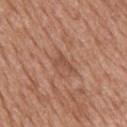<record>
<biopsy_status>not biopsied; imaged during a skin examination</biopsy_status>
<patient>
  <sex>male</sex>
  <age_approx>60</age_approx>
</patient>
<automated_metrics>
  <area_mm2_approx>3.0</area_mm2_approx>
  <eccentricity>0.85</eccentricity>
  <shape_asymmetry>0.5</shape_asymmetry>
  <border_irregularity_0_10>5.5</border_irregularity_0_10>
  <color_variation_0_10>0.0</color_variation_0_10>
  <peripheral_color_asymmetry>0.0</peripheral_color_asymmetry>
  <nevus_likeness_0_100>0</nevus_likeness_0_100>
  <lesion_detection_confidence_0_100>95</lesion_detection_confidence_0_100>
</automated_metrics>
<site>upper back</site>
<image>
  <source>total-body photography crop</source>
  <field_of_view_mm>15</field_of_view_mm>
</image>
<lighting>white-light</lighting>
<lesion_size>
  <long_diameter_mm_approx>2.5</long_diameter_mm_approx>
</lesion_size>
</record>